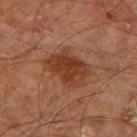workup: catalogued during a skin exam; not biopsied | site: the right leg | tile lighting: cross-polarized | subject: male, aged 58–62 | lesion size: about 5.5 mm | image: ~15 mm tile from a whole-body skin photo | automated metrics: an area of roughly 18 mm² and a shape-asymmetry score of about 0.25 (0 = symmetric); a lesion color around L≈38 a*≈24 b*≈31 in CIELAB, a lesion–skin lightness drop of about 9, and a normalized lesion–skin contrast near 8; a border-irregularity rating of about 3.5/10, a within-lesion color-variation index near 4/10, and a peripheral color-asymmetry measure near 1; an automated nevus-likeness rating near 80 out of 100 and a lesion-detection confidence of about 100/100.